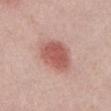– biopsy status · imaged on a skin check; not biopsied
– diameter · about 4 mm
– acquisition · ~15 mm crop, total-body skin-cancer survey
– patient · female, aged 38–42
– tile lighting · white-light
– TBP lesion metrics · a footprint of about 13 mm², an eccentricity of roughly 0.45, and a shape-asymmetry score of about 0.2 (0 = symmetric); border irregularity of about 2 on a 0–10 scale, internal color variation of about 3 on a 0–10 scale, and a peripheral color-asymmetry measure near 1
– anatomic site · the abdomen